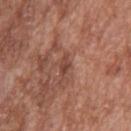This lesion was catalogued during total-body skin photography and was not selected for biopsy.